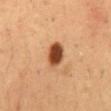Context:
Approximately 3.5 mm at its widest. A close-up tile cropped from a whole-body skin photograph, about 15 mm across. The patient is a male roughly 50 years of age. Located on the abdomen.